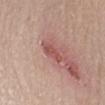notes: total-body-photography surveillance lesion; no biopsy
patient: female, aged approximately 60
site: the left forearm
image source: total-body-photography crop, ~15 mm field of view
illumination: white-light illumination
size: ≈3.5 mm